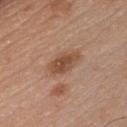<case>
  <automated_metrics>
    <area_mm2_approx>7.5</area_mm2_approx>
    <eccentricity>0.85</eccentricity>
    <border_irregularity_0_10>2.0</border_irregularity_0_10>
    <nevus_likeness_0_100>90</nevus_likeness_0_100>
    <lesion_detection_confidence_0_100>100</lesion_detection_confidence_0_100>
  </automated_metrics>
  <site>chest</site>
  <image>
    <source>total-body photography crop</source>
    <field_of_view_mm>15</field_of_view_mm>
  </image>
  <lesion_size>
    <long_diameter_mm_approx>4.0</long_diameter_mm_approx>
  </lesion_size>
  <patient>
    <sex>male</sex>
    <age_approx>45</age_approx>
  </patient>
  <lighting>white-light</lighting>
</case>